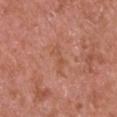{
  "biopsy_status": "not biopsied; imaged during a skin examination",
  "lighting": "white-light",
  "patient": {
    "sex": "male",
    "age_approx": 65
  },
  "image": {
    "source": "total-body photography crop",
    "field_of_view_mm": 15
  },
  "site": "left upper arm"
}